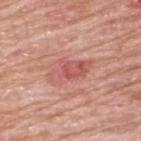Recorded during total-body skin imaging; not selected for excision or biopsy. A male patient, aged around 80. Located on the upper back. About 5 mm across. A close-up tile cropped from a whole-body skin photograph, about 15 mm across. The total-body-photography lesion software estimated a footprint of about 9.5 mm². The analysis additionally found a mean CIELAB color near L≈57 a*≈29 b*≈27 and a normalized lesion–skin contrast near 6. The analysis additionally found a nevus-likeness score of about 0/100 and lesion-presence confidence of about 100/100.